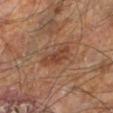Notes:
– follow-up · catalogued during a skin exam; not biopsied
– site · the right leg
– image · ~15 mm crop, total-body skin-cancer survey
– lighting · cross-polarized
– lesion size · ≈3.5 mm
– subject · male, aged 58–62